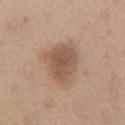| key | value |
|---|---|
| notes | catalogued during a skin exam; not biopsied |
| image source | 15 mm crop, total-body photography |
| patient | male, aged around 60 |
| site | the front of the torso |